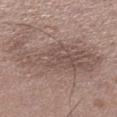{
  "biopsy_status": "not biopsied; imaged during a skin examination",
  "patient": {
    "sex": "male",
    "age_approx": 50
  },
  "image": {
    "source": "total-body photography crop",
    "field_of_view_mm": 15
  },
  "lighting": "white-light",
  "site": "left lower leg"
}